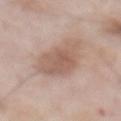<lesion>
  <biopsy_status>not biopsied; imaged during a skin examination</biopsy_status>
  <site>abdomen</site>
  <image>
    <source>total-body photography crop</source>
    <field_of_view_mm>15</field_of_view_mm>
  </image>
  <automated_metrics>
    <cielab_L>58</cielab_L>
    <cielab_a>18</cielab_a>
    <cielab_b>26</cielab_b>
    <vs_skin_darker_L>10.0</vs_skin_darker_L>
    <vs_skin_contrast_norm>7.0</vs_skin_contrast_norm>
    <border_irregularity_0_10>3.5</border_irregularity_0_10>
    <color_variation_0_10>3.0</color_variation_0_10>
    <peripheral_color_asymmetry>1.0</peripheral_color_asymmetry>
    <nevus_likeness_0_100>55</nevus_likeness_0_100>
    <lesion_detection_confidence_0_100>100</lesion_detection_confidence_0_100>
  </automated_metrics>
  <patient>
    <sex>male</sex>
    <age_approx>75</age_approx>
  </patient>
</lesion>